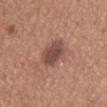Q: Is there a histopathology result?
A: imaged on a skin check; not biopsied
Q: What kind of image is this?
A: ~15 mm tile from a whole-body skin photo
Q: What are the patient's age and sex?
A: male, aged around 65
Q: What is the lesion's diameter?
A: ~5 mm (longest diameter)
Q: Illumination type?
A: white-light
Q: Lesion location?
A: the mid back